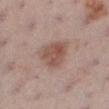| feature | finding |
|---|---|
| biopsy status | imaged on a skin check; not biopsied |
| automated lesion analysis | an eccentricity of roughly 0.4 and a symmetry-axis asymmetry near 0.25 |
| patient | female, aged around 55 |
| anatomic site | the left lower leg |
| imaging modality | 15 mm crop, total-body photography |
| illumination | white-light illumination |